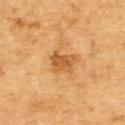follow-up: total-body-photography surveillance lesion; no biopsy
subject: male, aged around 85 or older
anatomic site: the upper back
TBP lesion metrics: an area of roughly 6 mm², an outline eccentricity of about 0.65 (0 = round, 1 = elongated), and a symmetry-axis asymmetry near 0.35; a lesion color around L≈48 a*≈22 b*≈40 in CIELAB, roughly 10 lightness units darker than nearby skin, and a normalized border contrast of about 7.5
lighting: cross-polarized
imaging modality: 15 mm crop, total-body photography